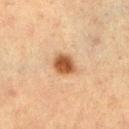Clinical impression: The lesion was tiled from a total-body skin photograph and was not biopsied. Background: This is a cross-polarized tile. The lesion is located on the leg. A female subject, aged 53–57. The recorded lesion diameter is about 3 mm. A 15 mm crop from a total-body photograph taken for skin-cancer surveillance.